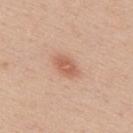biopsy status: catalogued during a skin exam; not biopsied | patient: male, roughly 35 years of age | diameter: about 3 mm | automated metrics: an area of roughly 4.5 mm², a shape eccentricity near 0.8, and two-axis asymmetry of about 0.25; a mean CIELAB color near L≈60 a*≈24 b*≈32 | acquisition: 15 mm crop, total-body photography | tile lighting: white-light | body site: the upper back.